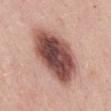This lesion was catalogued during total-body skin photography and was not selected for biopsy. Imaged with white-light lighting. Measured at roughly 8 mm in maximum diameter. The lesion is on the mid back. A male patient, roughly 25 years of age. A 15 mm close-up extracted from a 3D total-body photography capture.